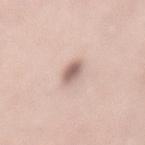follow-up=no biopsy performed (imaged during a skin exam)
lesion size=about 2.5 mm
lighting=white-light
site=the mid back
patient=female, aged 38 to 42
image source=total-body-photography crop, ~15 mm field of view
automated lesion analysis=two-axis asymmetry of about 0.15; a border-irregularity index near 1.5/10 and a within-lesion color-variation index near 3/10